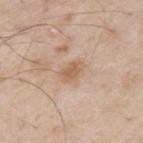Assessment: This lesion was catalogued during total-body skin photography and was not selected for biopsy. Clinical summary: The lesion is located on the left upper arm. About 3 mm across. The tile uses white-light illumination. This image is a 15 mm lesion crop taken from a total-body photograph. A male subject, aged around 65.